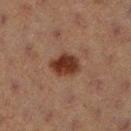{"biopsy_status": "not biopsied; imaged during a skin examination", "patient": {"sex": "female", "age_approx": 40}, "image": {"source": "total-body photography crop", "field_of_view_mm": 15}, "lighting": "cross-polarized", "site": "right lower leg", "lesion_size": {"long_diameter_mm_approx": 3.5}}